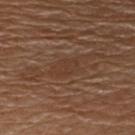  biopsy_status: not biopsied; imaged during a skin examination
  lesion_size:
    long_diameter_mm_approx: 3.0
  patient:
    sex: male
    age_approx: 70
  lighting: white-light
  site: front of the torso
  image:
    source: total-body photography crop
    field_of_view_mm: 15
  automated_metrics:
    border_irregularity_0_10: 3.5